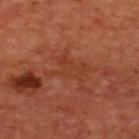This lesion was catalogued during total-body skin photography and was not selected for biopsy. A roughly 15 mm field-of-view crop from a total-body skin photograph. A male subject, aged 63–67. About 4.5 mm across. This is a cross-polarized tile. The lesion is located on the back. Automated image analysis of the tile measured a lesion area of about 8 mm² and a shape eccentricity near 0.75. And it measured a border-irregularity rating of about 7.5/10 and a within-lesion color-variation index near 2/10.